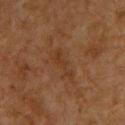The lesion was photographed on a routine skin check and not biopsied; there is no pathology result. The recorded lesion diameter is about 4 mm. A male patient, aged 58 to 62. From the front of the torso. Cropped from a whole-body photographic skin survey; the tile spans about 15 mm. Captured under cross-polarized illumination.